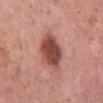Q: Is there a histopathology result?
A: no biopsy performed (imaged during a skin exam)
Q: What are the patient's age and sex?
A: female, in their 50s
Q: What is the imaging modality?
A: 15 mm crop, total-body photography
Q: What is the anatomic site?
A: the right lower leg
Q: Illumination type?
A: white-light illumination
Q: Automated lesion metrics?
A: a footprint of about 13 mm² and an eccentricity of roughly 0.5; an average lesion color of about L≈48 a*≈26 b*≈26 (CIELAB) and a lesion–skin lightness drop of about 16; a peripheral color-asymmetry measure near 1; an automated nevus-likeness rating near 65 out of 100 and a detector confidence of about 100 out of 100 that the crop contains a lesion
Q: How large is the lesion?
A: ≈4.5 mm